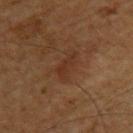patient:
  sex: male
  age_approx: 60
site: right upper arm
image:
  source: total-body photography crop
  field_of_view_mm: 15
automated_metrics:
  cielab_L: 28
  cielab_a: 19
  cielab_b: 27
  vs_skin_contrast_norm: 6.0
  border_irregularity_0_10: 4.5
  color_variation_0_10: 1.0
  peripheral_color_asymmetry: 0.0
  nevus_likeness_0_100: 0
  lesion_detection_confidence_0_100: 100